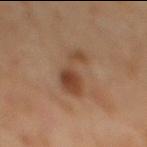Assessment:
No biopsy was performed on this lesion — it was imaged during a full skin examination and was not determined to be concerning.
Context:
Located on the mid back. A close-up tile cropped from a whole-body skin photograph, about 15 mm across. An algorithmic analysis of the crop reported a border-irregularity index near 7/10, a within-lesion color-variation index near 3.5/10, and a peripheral color-asymmetry measure near 1. About 5.5 mm across. Captured under cross-polarized illumination. A male subject, aged around 60.